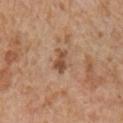workup = no biopsy performed (imaged during a skin exam); body site = the right upper arm; acquisition = ~15 mm tile from a whole-body skin photo; patient = male, aged around 65.